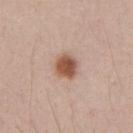Q: Is there a histopathology result?
A: total-body-photography surveillance lesion; no biopsy
Q: What kind of image is this?
A: ~15 mm crop, total-body skin-cancer survey
Q: Patient demographics?
A: male, in their 40s
Q: Lesion location?
A: the chest